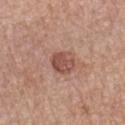The lesion was tiled from a total-body skin photograph and was not biopsied. On the left forearm. Approximately 3 mm at its widest. Captured under white-light illumination. A close-up tile cropped from a whole-body skin photograph, about 15 mm across. A female patient in their 60s.